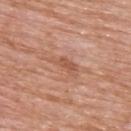Clinical impression: Part of a total-body skin-imaging series; this lesion was reviewed on a skin check and was not flagged for biopsy. Acquisition and patient details: This is a white-light tile. Longest diameter approximately 4 mm. Cropped from a total-body skin-imaging series; the visible field is about 15 mm. A female subject, aged approximately 70. The lesion is located on the upper back.